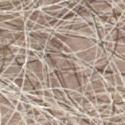The lesion was tiled from a total-body skin photograph and was not biopsied. A 15 mm crop from a total-body photograph taken for skin-cancer surveillance. The lesion is on the chest. A male subject, approximately 55 years of age.